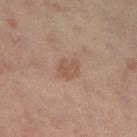Clinical impression: No biopsy was performed on this lesion — it was imaged during a full skin examination and was not determined to be concerning. Clinical summary: Approximately 2.5 mm at its widest. Cropped from a whole-body photographic skin survey; the tile spans about 15 mm. The lesion is located on the leg. A female subject about 65 years old. The tile uses cross-polarized illumination.